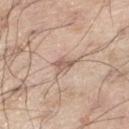{"biopsy_status": "not biopsied; imaged during a skin examination", "lighting": "white-light", "site": "right thigh", "image": {"source": "total-body photography crop", "field_of_view_mm": 15}, "lesion_size": {"long_diameter_mm_approx": 2.5}, "patient": {"sex": "male", "age_approx": 70}}